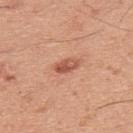| key | value |
|---|---|
| biopsy status | total-body-photography surveillance lesion; no biopsy |
| image | 15 mm crop, total-body photography |
| diameter | ~3 mm (longest diameter) |
| tile lighting | white-light illumination |
| patient | male, approximately 45 years of age |
| site | the upper back |